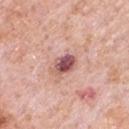Assessment:
Part of a total-body skin-imaging series; this lesion was reviewed on a skin check and was not flagged for biopsy.
Acquisition and patient details:
A 15 mm close-up tile from a total-body photography series done for melanoma screening. The lesion is on the chest. The subject is a male roughly 80 years of age. Approximately 3.5 mm at its widest.